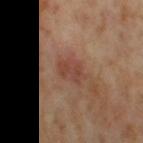The lesion was photographed on a routine skin check and not biopsied; there is no pathology result.
Imaged with cross-polarized lighting.
The lesion's longest dimension is about 3 mm.
On the right thigh.
The subject is a female approximately 55 years of age.
A 15 mm close-up tile from a total-body photography series done for melanoma screening.